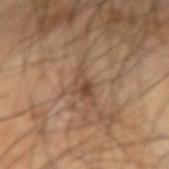Findings:
– follow-up · imaged on a skin check; not biopsied
– lighting · cross-polarized
– patient · male, aged around 65
– location · the right forearm
– TBP lesion metrics · a lesion area of about 5.5 mm², an eccentricity of roughly 0.9, and a symmetry-axis asymmetry near 0.5; a lesion color around L≈47 a*≈17 b*≈30 in CIELAB, roughly 8 lightness units darker than nearby skin, and a normalized lesion–skin contrast near 6; a border-irregularity rating of about 7/10, a color-variation rating of about 5.5/10, and peripheral color asymmetry of about 2; a classifier nevus-likeness of about 0/100 and a lesion-detection confidence of about 70/100
– acquisition · ~15 mm tile from a whole-body skin photo